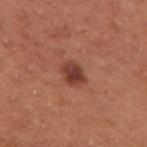Clinical summary: Located on the right upper arm. Cropped from a whole-body photographic skin survey; the tile spans about 15 mm. A female patient roughly 65 years of age. The recorded lesion diameter is about 3 mm. Captured under white-light illumination. The lesion-visualizer software estimated a shape eccentricity near 0.7 and a symmetry-axis asymmetry near 0.2. The software also gave a lesion color around L≈40 a*≈26 b*≈27 in CIELAB and a lesion–skin lightness drop of about 12. It also reported a border-irregularity index near 2/10, internal color variation of about 3.5 on a 0–10 scale, and a peripheral color-asymmetry measure near 1. The software also gave a detector confidence of about 100 out of 100 that the crop contains a lesion.